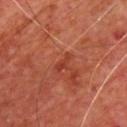{"biopsy_status": "not biopsied; imaged during a skin examination", "site": "front of the torso", "lesion_size": {"long_diameter_mm_approx": 2.5}, "lighting": "cross-polarized", "image": {"source": "total-body photography crop", "field_of_view_mm": 15}, "patient": {"sex": "male", "age_approx": 65}}